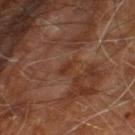| field | value |
|---|---|
| follow-up | no biopsy performed (imaged during a skin exam) |
| location | the right leg |
| lesion diameter | about 2.5 mm |
| image source | ~15 mm tile from a whole-body skin photo |
| lighting | cross-polarized illumination |
| subject | male, in their 60s |
| TBP lesion metrics | border irregularity of about 3.5 on a 0–10 scale, a color-variation rating of about 0.5/10, and a peripheral color-asymmetry measure near 0; an automated nevus-likeness rating near 0 out of 100 |